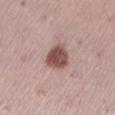Captured during whole-body skin photography for melanoma surveillance; the lesion was not biopsied.